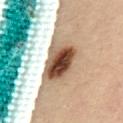Q: Is there a histopathology result?
A: imaged on a skin check; not biopsied
Q: How was the tile lit?
A: cross-polarized
Q: What is the lesion's diameter?
A: ~6 mm (longest diameter)
Q: What is the anatomic site?
A: the abdomen
Q: What is the imaging modality?
A: ~15 mm crop, total-body skin-cancer survey